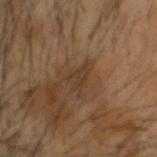| field | value |
|---|---|
| notes | imaged on a skin check; not biopsied |
| acquisition | ~15 mm crop, total-body skin-cancer survey |
| location | the head or neck |
| tile lighting | cross-polarized |
| subject | male, roughly 55 years of age |